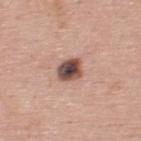biopsy_status: not biopsied; imaged during a skin examination
lesion_size:
  long_diameter_mm_approx: 3.0
image:
  source: total-body photography crop
  field_of_view_mm: 15
site: upper back
lighting: white-light
patient:
  sex: male
  age_approx: 65